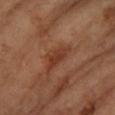Cropped from a total-body skin-imaging series; the visible field is about 15 mm. The lesion is on the left forearm. The patient is a female aged around 60. This is a cross-polarized tile. The lesion's longest dimension is about 4 mm.